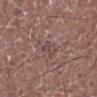Clinical summary:
Imaged with white-light lighting. The subject is a male approximately 75 years of age. About 2.5 mm across. A 15 mm close-up extracted from a 3D total-body photography capture. The total-body-photography lesion software estimated a footprint of about 3 mm², a shape eccentricity near 0.85, and a symmetry-axis asymmetry near 0.35. And it measured a mean CIELAB color near L≈45 a*≈19 b*≈19, roughly 6 lightness units darker than nearby skin, and a lesion-to-skin contrast of about 5 (normalized; higher = more distinct). The software also gave a border-irregularity rating of about 3/10 and a color-variation rating of about 2.5/10. The analysis additionally found a classifier nevus-likeness of about 0/100 and lesion-presence confidence of about 80/100. The lesion is on the leg.